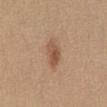{"biopsy_status": "not biopsied; imaged during a skin examination", "lesion_size": {"long_diameter_mm_approx": 3.5}, "site": "abdomen", "image": {"source": "total-body photography crop", "field_of_view_mm": 15}, "automated_metrics": {"area_mm2_approx": 5.5, "eccentricity": 0.8, "shape_asymmetry": 0.25, "border_irregularity_0_10": 2.5, "color_variation_0_10": 4.0, "peripheral_color_asymmetry": 1.5, "lesion_detection_confidence_0_100": 100}, "patient": {"sex": "female", "age_approx": 35}}